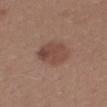Notes:
– notes — catalogued during a skin exam; not biopsied
– patient — female, aged around 25
– diameter — about 4.5 mm
– image — total-body-photography crop, ~15 mm field of view
– tile lighting — white-light illumination
– anatomic site — the mid back